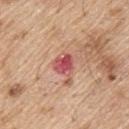Imaged during a routine full-body skin examination; the lesion was not biopsied and no histopathology is available. A 15 mm close-up extracted from a 3D total-body photography capture. The lesion is located on the back. A male subject, aged 68–72. Captured under white-light illumination. The total-body-photography lesion software estimated an area of roughly 5 mm² and an outline eccentricity of about 0.4 (0 = round, 1 = elongated). The analysis additionally found a lesion color around L≈53 a*≈34 b*≈24 in CIELAB, about 13 CIELAB-L* units darker than the surrounding skin, and a normalized lesion–skin contrast near 10. The software also gave a border-irregularity index near 2.5/10 and internal color variation of about 4.5 on a 0–10 scale. The recorded lesion diameter is about 2.5 mm.